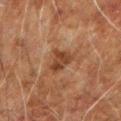{
  "biopsy_status": "not biopsied; imaged during a skin examination",
  "image": {
    "source": "total-body photography crop",
    "field_of_view_mm": 15
  },
  "patient": {
    "sex": "male",
    "age_approx": 60
  },
  "lighting": "cross-polarized",
  "lesion_size": {
    "long_diameter_mm_approx": 3.5
  },
  "site": "left arm"
}